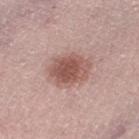Clinical impression:
Captured during whole-body skin photography for melanoma surveillance; the lesion was not biopsied.
Image and clinical context:
A close-up tile cropped from a whole-body skin photograph, about 15 mm across. An algorithmic analysis of the crop reported a lesion area of about 12 mm², an outline eccentricity of about 0.6 (0 = round, 1 = elongated), and a shape-asymmetry score of about 0.15 (0 = symmetric). The software also gave an average lesion color of about L≈54 a*≈22 b*≈24 (CIELAB). The analysis additionally found a border-irregularity index near 2/10, internal color variation of about 4 on a 0–10 scale, and radial color variation of about 1.5. Imaged with white-light lighting. A female patient, aged 58 to 62. About 4.5 mm across. From the right thigh.